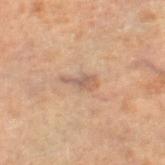notes: no biopsy performed (imaged during a skin exam); diameter: ≈3.5 mm; illumination: cross-polarized; imaging modality: total-body-photography crop, ~15 mm field of view; anatomic site: the right lower leg; patient: male, aged around 70.